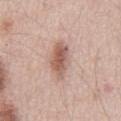notes = catalogued during a skin exam; not biopsied | imaging modality = total-body-photography crop, ~15 mm field of view | illumination = white-light illumination | body site = the chest | subject = male, aged 53–57.